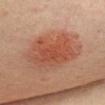{"biopsy_status": "not biopsied; imaged during a skin examination"}